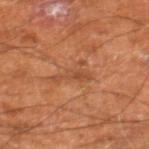Case summary:
- notes — no biopsy performed (imaged during a skin exam)
- diameter — ~4 mm (longest diameter)
- TBP lesion metrics — a mean CIELAB color near L≈47 a*≈25 b*≈34, roughly 7 lightness units darker than nearby skin, and a normalized border contrast of about 5
- subject — male, aged 58–62
- acquisition — ~15 mm crop, total-body skin-cancer survey
- location — the right leg